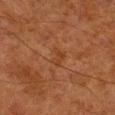The lesion is located on the right lower leg.
The subject is a male aged around 80.
This image is a 15 mm lesion crop taken from a total-body photograph.
Captured under cross-polarized illumination.
The lesion's longest dimension is about 3 mm.